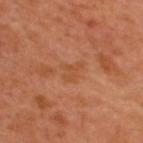Notes:
* patient: male, in their 50s
* anatomic site: the upper back
* diameter: ~2.5 mm (longest diameter)
* acquisition: ~15 mm crop, total-body skin-cancer survey
* tile lighting: cross-polarized